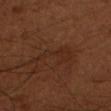Q: Is there a histopathology result?
A: imaged on a skin check; not biopsied
Q: How was the tile lit?
A: cross-polarized illumination
Q: What did automated image analysis measure?
A: a lesion area of about 6 mm²; a lesion color around L≈26 a*≈19 b*≈26 in CIELAB, about 4 CIELAB-L* units darker than the surrounding skin, and a normalized lesion–skin contrast near 5; border irregularity of about 8.5 on a 0–10 scale, a color-variation rating of about 1.5/10, and radial color variation of about 0.5; an automated nevus-likeness rating near 0 out of 100 and a lesion-detection confidence of about 95/100
Q: Who is the patient?
A: male, aged 48–52
Q: What kind of image is this?
A: total-body-photography crop, ~15 mm field of view
Q: What is the anatomic site?
A: the arm